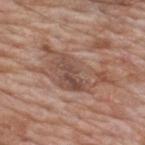Q: Is there a histopathology result?
A: catalogued during a skin exam; not biopsied
Q: What is the anatomic site?
A: the upper back
Q: What did automated image analysis measure?
A: an average lesion color of about L≈50 a*≈19 b*≈26 (CIELAB), about 9 CIELAB-L* units darker than the surrounding skin, and a normalized border contrast of about 6.5; a border-irregularity rating of about 6/10, a within-lesion color-variation index near 5/10, and radial color variation of about 1.5
Q: What are the patient's age and sex?
A: male, approximately 60 years of age
Q: Illumination type?
A: white-light illumination
Q: What is the imaging modality?
A: ~15 mm tile from a whole-body skin photo